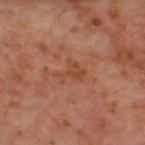Case summary:
– biopsy status: catalogued during a skin exam; not biopsied
– imaging modality: total-body-photography crop, ~15 mm field of view
– site: the upper back
– patient: male, in their 60s